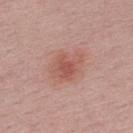Clinical impression:
This lesion was catalogued during total-body skin photography and was not selected for biopsy.
Image and clinical context:
Imaged with white-light lighting. The recorded lesion diameter is about 4.5 mm. The subject is a male aged 28–32. The lesion-visualizer software estimated an average lesion color of about L≈56 a*≈24 b*≈26 (CIELAB) and a normalized border contrast of about 6. The software also gave a border-irregularity index near 2/10 and a color-variation rating of about 4/10. It also reported a classifier nevus-likeness of about 55/100 and a lesion-detection confidence of about 100/100. A 15 mm crop from a total-body photograph taken for skin-cancer surveillance.